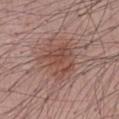Impression: This lesion was catalogued during total-body skin photography and was not selected for biopsy. Clinical summary: Measured at roughly 5.5 mm in maximum diameter. The patient is a male in their 40s. The lesion-visualizer software estimated a footprint of about 19 mm², an eccentricity of roughly 0.5, and a shape-asymmetry score of about 0.3 (0 = symmetric). It also reported an average lesion color of about L≈49 a*≈21 b*≈25 (CIELAB) and about 8 CIELAB-L* units darker than the surrounding skin. Cropped from a total-body skin-imaging series; the visible field is about 15 mm. The lesion is on the front of the torso. This is a white-light tile.